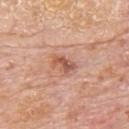The lesion was tiled from a total-body skin photograph and was not biopsied.
The lesion is located on the back.
A region of skin cropped from a whole-body photographic capture, roughly 15 mm wide.
A male subject, aged approximately 80.